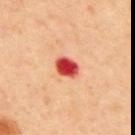Part of a total-body skin-imaging series; this lesion was reviewed on a skin check and was not flagged for biopsy.
A 15 mm close-up extracted from a 3D total-body photography capture.
The lesion is on the back.
Imaged with cross-polarized lighting.
A male subject aged approximately 65.